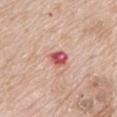Case summary:
- image source — total-body-photography crop, ~15 mm field of view
- lighting — white-light illumination
- anatomic site — the mid back
- subject — male, aged around 65